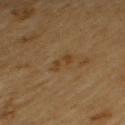Findings:
* body site · the upper back
* image source · ~15 mm crop, total-body skin-cancer survey
* lighting · cross-polarized illumination
* patient · male, in their mid-80s or older
* lesion diameter · ≈3 mm